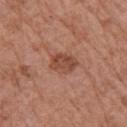workup — no biopsy performed (imaged during a skin exam)
subject — female, about 75 years old
lighting — white-light
TBP lesion metrics — a footprint of about 7 mm², an outline eccentricity of about 0.65 (0 = round, 1 = elongated), and a symmetry-axis asymmetry near 0.2; an average lesion color of about L≈47 a*≈24 b*≈29 (CIELAB), roughly 10 lightness units darker than nearby skin, and a normalized lesion–skin contrast near 7; border irregularity of about 2 on a 0–10 scale and peripheral color asymmetry of about 1; a classifier nevus-likeness of about 45/100 and lesion-presence confidence of about 100/100
acquisition — ~15 mm tile from a whole-body skin photo
site — the right upper arm